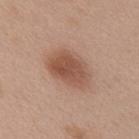| field | value |
|---|---|
| illumination | white-light illumination |
| anatomic site | the front of the torso |
| lesion diameter | ~5 mm (longest diameter) |
| patient | female, aged 38–42 |
| imaging modality | total-body-photography crop, ~15 mm field of view |